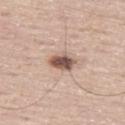biopsy status = total-body-photography surveillance lesion; no biopsy
subject = male, in their mid-60s
image = ~15 mm tile from a whole-body skin photo
anatomic site = the left thigh